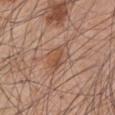notes: no biopsy performed (imaged during a skin exam); location: the arm; subject: male, aged approximately 45; image source: ~15 mm tile from a whole-body skin photo.